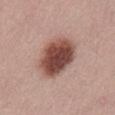Recorded during total-body skin imaging; not selected for excision or biopsy.
Approximately 5 mm at its widest.
An algorithmic analysis of the crop reported a footprint of about 19 mm². And it measured border irregularity of about 1.5 on a 0–10 scale, internal color variation of about 6 on a 0–10 scale, and radial color variation of about 1.5. It also reported an automated nevus-likeness rating near 95 out of 100.
The lesion is located on the lower back.
A male patient aged approximately 75.
A roughly 15 mm field-of-view crop from a total-body skin photograph.
Captured under white-light illumination.